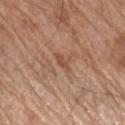site: arm
lighting: white-light
patient:
  sex: male
  age_approx: 70
image:
  source: total-body photography crop
  field_of_view_mm: 15